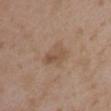Case summary:
- workup: imaged on a skin check; not biopsied
- automated lesion analysis: a mean CIELAB color near L≈51 a*≈17 b*≈29, a lesion–skin lightness drop of about 7, and a normalized border contrast of about 6; a border-irregularity index near 3.5/10 and a peripheral color-asymmetry measure near 1.5
- patient: female, aged 33 to 37
- illumination: white-light illumination
- acquisition: total-body-photography crop, ~15 mm field of view
- body site: the left upper arm